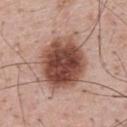workup: imaged on a skin check; not biopsied
tile lighting: white-light
image: ~15 mm tile from a whole-body skin photo
patient: male, in their mid- to late 50s
anatomic site: the upper back
size: ≈6.5 mm